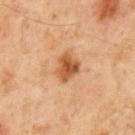• biopsy status — total-body-photography surveillance lesion; no biopsy
• patient — male, roughly 60 years of age
• body site — the mid back
• image source — ~15 mm tile from a whole-body skin photo